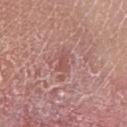Case summary:
- workup · total-body-photography surveillance lesion; no biopsy
- illumination · white-light
- diameter · about 2.5 mm
- site · the left lower leg
- subject · male, aged 78 to 82
- acquisition · ~15 mm tile from a whole-body skin photo